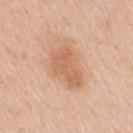This lesion was catalogued during total-body skin photography and was not selected for biopsy.
A male patient, in their 50s.
A region of skin cropped from a whole-body photographic capture, roughly 15 mm wide.
The lesion is on the arm.
The tile uses white-light illumination.
The total-body-photography lesion software estimated an area of roughly 13 mm², an eccentricity of roughly 0.8, and two-axis asymmetry of about 0.3. It also reported a lesion–skin lightness drop of about 9 and a lesion-to-skin contrast of about 6.5 (normalized; higher = more distinct). The analysis additionally found an automated nevus-likeness rating near 60 out of 100 and lesion-presence confidence of about 100/100.
Measured at roughly 5 mm in maximum diameter.